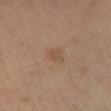Assessment:
Imaged during a routine full-body skin examination; the lesion was not biopsied and no histopathology is available.
Context:
Imaged with cross-polarized lighting. The lesion is located on the leg. A male patient, approximately 60 years of age. A region of skin cropped from a whole-body photographic capture, roughly 15 mm wide. Approximately 3 mm at its widest. The lesion-visualizer software estimated a mean CIELAB color near L≈45 a*≈14 b*≈27 and about 5 CIELAB-L* units darker than the surrounding skin. And it measured a border-irregularity index near 2/10 and a color-variation rating of about 2/10.